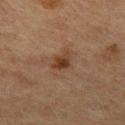Assessment:
Captured during whole-body skin photography for melanoma surveillance; the lesion was not biopsied.